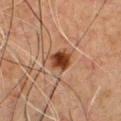This lesion was catalogued during total-body skin photography and was not selected for biopsy. From the chest. A lesion tile, about 15 mm wide, cut from a 3D total-body photograph. A male patient in their 50s.